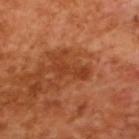Q: What are the patient's age and sex?
A: male, aged approximately 65
Q: How was this image acquired?
A: ~15 mm tile from a whole-body skin photo
Q: Lesion size?
A: about 4.5 mm